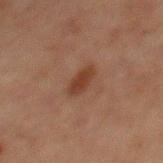Cropped from a total-body skin-imaging series; the visible field is about 15 mm. A male patient aged around 65. The lesion's longest dimension is about 3.5 mm. Imaged with cross-polarized lighting. An algorithmic analysis of the crop reported an eccentricity of roughly 0.9 and two-axis asymmetry of about 0.3. The software also gave an average lesion color of about L≈30 a*≈19 b*≈25 (CIELAB) and a normalized lesion–skin contrast near 8.5. The analysis additionally found radial color variation of about 0.5. The lesion is on the abdomen.